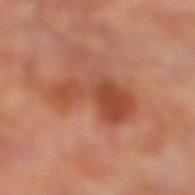notes: imaged on a skin check; not biopsied
diameter: about 6.5 mm
automated lesion analysis: an area of roughly 17 mm², a shape eccentricity near 0.85, and two-axis asymmetry of about 0.45; a mean CIELAB color near L≈47 a*≈28 b*≈33, roughly 9 lightness units darker than nearby skin, and a normalized lesion–skin contrast near 7; border irregularity of about 5.5 on a 0–10 scale and radial color variation of about 1.5; a classifier nevus-likeness of about 0/100 and a lesion-detection confidence of about 100/100
image source: total-body-photography crop, ~15 mm field of view
site: the right lower leg
illumination: cross-polarized
patient: male, aged approximately 70